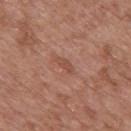Impression:
Captured during whole-body skin photography for melanoma surveillance; the lesion was not biopsied.
Background:
A male subject, in their 50s. A region of skin cropped from a whole-body photographic capture, roughly 15 mm wide. Imaged with white-light lighting. The total-body-photography lesion software estimated about 7 CIELAB-L* units darker than the surrounding skin and a normalized border contrast of about 5.5. Measured at roughly 2.5 mm in maximum diameter. Located on the mid back.